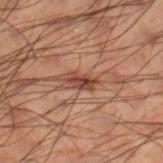{"biopsy_status": "not biopsied; imaged during a skin examination", "patient": {"sex": "male", "age_approx": 50}, "lesion_size": {"long_diameter_mm_approx": 3.0}, "site": "right lower leg", "image": {"source": "total-body photography crop", "field_of_view_mm": 15}, "automated_metrics": {"eccentricity": 0.85, "shape_asymmetry": 0.3, "cielab_L": 33, "cielab_a": 19, "cielab_b": 23, "vs_skin_contrast_norm": 9.0, "border_irregularity_0_10": 3.5, "color_variation_0_10": 4.0, "peripheral_color_asymmetry": 1.5}}